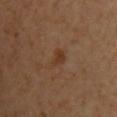A region of skin cropped from a whole-body photographic capture, roughly 15 mm wide. A female subject approximately 40 years of age. On the chest. This is a cross-polarized tile. Longest diameter approximately 2.5 mm.